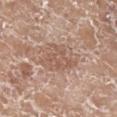The lesion was tiled from a total-body skin photograph and was not biopsied.
A female patient, about 75 years old.
Imaged with white-light lighting.
The recorded lesion diameter is about 5.5 mm.
Cropped from a whole-body photographic skin survey; the tile spans about 15 mm.
The lesion is on the left lower leg.